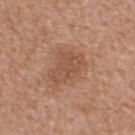  biopsy_status: not biopsied; imaged during a skin examination
  lighting: white-light
  site: back
  lesion_size:
    long_diameter_mm_approx: 4.5
  image:
    source: total-body photography crop
    field_of_view_mm: 15
  automated_metrics:
    cielab_L: 51
    cielab_a: 22
    cielab_b: 30
    vs_skin_darker_L: 8.0
    vs_skin_contrast_norm: 5.5
    nevus_likeness_0_100: 45
    lesion_detection_confidence_0_100: 100
  patient:
    sex: male
    age_approx: 55